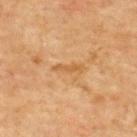Acquisition and patient details:
The lesion is located on the upper back. The total-body-photography lesion software estimated a lesion area of about 2 mm², an outline eccentricity of about 0.95 (0 = round, 1 = elongated), and two-axis asymmetry of about 0.4. It also reported about 8 CIELAB-L* units darker than the surrounding skin and a normalized border contrast of about 6.5. The analysis additionally found a nevus-likeness score of about 0/100 and a detector confidence of about 95 out of 100 that the crop contains a lesion. About 3 mm across. The patient is a male aged 68–72. Cropped from a whole-body photographic skin survey; the tile spans about 15 mm.